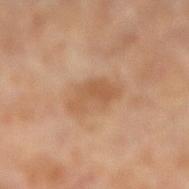Impression:
The lesion was photographed on a routine skin check and not biopsied; there is no pathology result.
Acquisition and patient details:
This is a cross-polarized tile. The patient is a female about 70 years old. Located on the leg. The lesion's longest dimension is about 4.5 mm. A roughly 15 mm field-of-view crop from a total-body skin photograph.